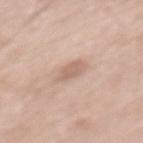Imaged during a routine full-body skin examination; the lesion was not biopsied and no histopathology is available.
A male subject, approximately 70 years of age.
Captured under white-light illumination.
A roughly 15 mm field-of-view crop from a total-body skin photograph.
Located on the mid back.
Automated tile analysis of the lesion measured an eccentricity of roughly 0.8 and a symmetry-axis asymmetry near 0.25. The software also gave an average lesion color of about L≈63 a*≈17 b*≈27 (CIELAB), a lesion–skin lightness drop of about 9, and a normalized lesion–skin contrast near 5.5.
Approximately 3 mm at its widest.